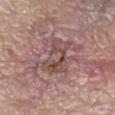notes: no biopsy performed (imaged during a skin exam)
site: the right lower leg
patient: female, roughly 85 years of age or older
imaging modality: ~15 mm crop, total-body skin-cancer survey
illumination: white-light
size: ~5.5 mm (longest diameter)